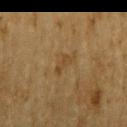Captured during whole-body skin photography for melanoma surveillance; the lesion was not biopsied. On the right upper arm. A 15 mm crop from a total-body photograph taken for skin-cancer surveillance. An algorithmic analysis of the crop reported a lesion area of about 3 mm², a shape eccentricity near 0.9, and a symmetry-axis asymmetry near 0.4. This is a cross-polarized tile. The patient is a male aged approximately 85 or older.